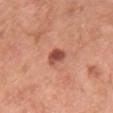The lesion was tiled from a total-body skin photograph and was not biopsied.
An algorithmic analysis of the crop reported a lesion color around L≈51 a*≈28 b*≈31 in CIELAB, roughly 14 lightness units darker than nearby skin, and a normalized lesion–skin contrast near 9.5. It also reported a border-irregularity rating of about 1.5/10, internal color variation of about 3.5 on a 0–10 scale, and peripheral color asymmetry of about 1.
The lesion is located on the chest.
Measured at roughly 2.5 mm in maximum diameter.
A 15 mm close-up tile from a total-body photography series done for melanoma screening.
The tile uses white-light illumination.
A male patient, aged around 60.